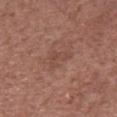notes=no biopsy performed (imaged during a skin exam)
subject=male, in their 60s
image=~15 mm tile from a whole-body skin photo
location=the front of the torso